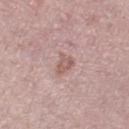A 15 mm close-up extracted from a 3D total-body photography capture. From the leg. A female subject in their 40s. About 3 mm across.